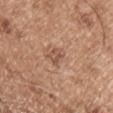A male patient, roughly 55 years of age. A 15 mm crop from a total-body photograph taken for skin-cancer surveillance. The lesion is on the left upper arm.